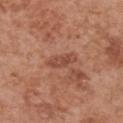workup: total-body-photography surveillance lesion; no biopsy
lesion size: ~3.5 mm (longest diameter)
image source: 15 mm crop, total-body photography
subject: female, aged approximately 65
lighting: white-light
site: the chest
TBP lesion metrics: a lesion area of about 4 mm² and a shape-asymmetry score of about 0.3 (0 = symmetric); a lesion color around L≈48 a*≈24 b*≈29 in CIELAB and a normalized border contrast of about 6.5; a within-lesion color-variation index near 2.5/10